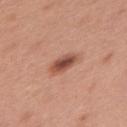  biopsy_status: not biopsied; imaged during a skin examination
  site: arm
  image:
    source: total-body photography crop
    field_of_view_mm: 15
  lesion_size:
    long_diameter_mm_approx: 3.5
  lighting: white-light
  patient:
    sex: female
    age_approx: 50